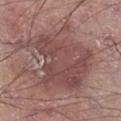Assessment: Captured during whole-body skin photography for melanoma surveillance; the lesion was not biopsied. Background: Imaged with white-light lighting. Cropped from a whole-body photographic skin survey; the tile spans about 15 mm. Longest diameter approximately 9 mm. On the right lower leg. A male patient aged around 70.